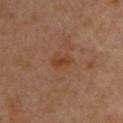Notes:
* biopsy status — no biopsy performed (imaged during a skin exam)
* patient — female, aged 58 to 62
* location — the upper back
* acquisition — ~15 mm tile from a whole-body skin photo
* automated lesion analysis — a mean CIELAB color near L≈42 a*≈23 b*≈33 and a lesion–skin lightness drop of about 6; a border-irregularity rating of about 2.5/10, internal color variation of about 2 on a 0–10 scale, and peripheral color asymmetry of about 0.5; a lesion-detection confidence of about 100/100
* lighting — cross-polarized